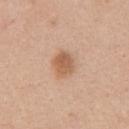Q: Lesion location?
A: the chest
Q: Who is the patient?
A: female, aged around 45
Q: Illumination type?
A: white-light illumination
Q: What kind of image is this?
A: ~15 mm crop, total-body skin-cancer survey
Q: What is the lesion's diameter?
A: ≈3 mm
Q: What did automated image analysis measure?
A: a footprint of about 7 mm², a shape eccentricity near 0.6, and a symmetry-axis asymmetry near 0.15; border irregularity of about 1.5 on a 0–10 scale and peripheral color asymmetry of about 1